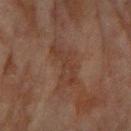workup = no biopsy performed (imaged during a skin exam); anatomic site = the arm; image = 15 mm crop, total-body photography; subject = female, in their 80s.